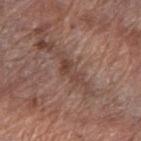Notes:
* notes · no biopsy performed (imaged during a skin exam)